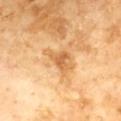The lesion was photographed on a routine skin check and not biopsied; there is no pathology result.
A lesion tile, about 15 mm wide, cut from a 3D total-body photograph.
The patient is a male approximately 60 years of age.
Imaged with cross-polarized lighting.
Automated image analysis of the tile measured a footprint of about 7 mm², an outline eccentricity of about 0.75 (0 = round, 1 = elongated), and a symmetry-axis asymmetry near 0.4. The analysis additionally found a lesion–skin lightness drop of about 10. The analysis additionally found border irregularity of about 4.5 on a 0–10 scale, a within-lesion color-variation index near 4/10, and peripheral color asymmetry of about 1. It also reported an automated nevus-likeness rating near 0 out of 100 and a lesion-detection confidence of about 100/100.
From the chest.